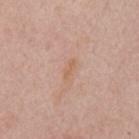| feature | finding |
|---|---|
| biopsy status | no biopsy performed (imaged during a skin exam) |
| image source | ~15 mm tile from a whole-body skin photo |
| tile lighting | white-light illumination |
| site | the mid back |
| patient | male, aged around 55 |